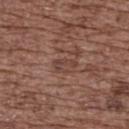Findings:
* biopsy status · no biopsy performed (imaged during a skin exam)
* imaging modality · 15 mm crop, total-body photography
* subject · female, aged 73–77
* diameter · ≈3 mm
* location · the back